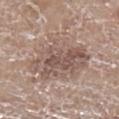Clinical summary: The lesion-visualizer software estimated an area of roughly 30 mm², a shape eccentricity near 0.55, and a shape-asymmetry score of about 0.25 (0 = symmetric). And it measured border irregularity of about 4 on a 0–10 scale, a within-lesion color-variation index near 6/10, and radial color variation of about 2. It also reported an automated nevus-likeness rating near 0 out of 100 and a detector confidence of about 90 out of 100 that the crop contains a lesion. Located on the left lower leg. Cropped from a total-body skin-imaging series; the visible field is about 15 mm. The patient is a female roughly 75 years of age. About 7.5 mm across. This is a white-light tile.